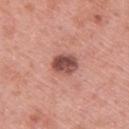{"image": {"source": "total-body photography crop", "field_of_view_mm": 15}, "automated_metrics": {"area_mm2_approx": 7.0, "eccentricity": 0.55, "shape_asymmetry": 0.2, "cielab_L": 50, "cielab_a": 25, "cielab_b": 25, "vs_skin_darker_L": 15.0, "vs_skin_contrast_norm": 10.0}, "patient": {"sex": "male", "age_approx": 60}, "site": "upper back", "lighting": "white-light"}